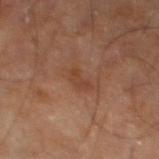follow-up=no biopsy performed (imaged during a skin exam); illumination=cross-polarized; site=the right leg; lesion size=~3 mm (longest diameter); imaging modality=~15 mm crop, total-body skin-cancer survey; subject=male, aged 58–62.